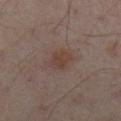A 15 mm crop from a total-body photograph taken for skin-cancer surveillance. The subject is a male in their 50s. Captured under cross-polarized illumination. Automated image analysis of the tile measured a lesion area of about 5.5 mm², a shape eccentricity near 0.65, and a symmetry-axis asymmetry near 0.25. And it measured an automated nevus-likeness rating near 20 out of 100 and a lesion-detection confidence of about 100/100. From the front of the torso.